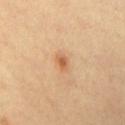| field | value |
|---|---|
| workup | imaged on a skin check; not biopsied |